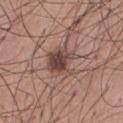Q: Is there a histopathology result?
A: no biopsy performed (imaged during a skin exam)
Q: Who is the patient?
A: male, about 30 years old
Q: How was this image acquired?
A: ~15 mm tile from a whole-body skin photo
Q: Where on the body is the lesion?
A: the front of the torso